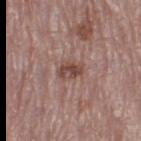The lesion was photographed on a routine skin check and not biopsied; there is no pathology result. A 15 mm close-up tile from a total-body photography series done for melanoma screening. About 3 mm across. Automated tile analysis of the lesion measured an automated nevus-likeness rating near 0 out of 100 and a detector confidence of about 100 out of 100 that the crop contains a lesion. The lesion is on the right thigh. The tile uses white-light illumination. A female patient, about 65 years old.